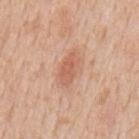notes: total-body-photography surveillance lesion; no biopsy
tile lighting: white-light illumination
patient: male, in their mid-60s
location: the mid back
diameter: about 4 mm
image: ~15 mm tile from a whole-body skin photo